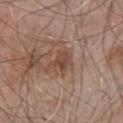Q: Was this lesion biopsied?
A: total-body-photography surveillance lesion; no biopsy
Q: What is the anatomic site?
A: the chest
Q: Illumination type?
A: white-light
Q: Patient demographics?
A: male, approximately 80 years of age
Q: How was this image acquired?
A: total-body-photography crop, ~15 mm field of view
Q: Automated lesion metrics?
A: a footprint of about 4 mm², an eccentricity of roughly 0.55, and two-axis asymmetry of about 0.35; border irregularity of about 4 on a 0–10 scale and a peripheral color-asymmetry measure near 1; a classifier nevus-likeness of about 20/100
Q: Lesion size?
A: ~2.5 mm (longest diameter)